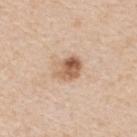Findings:
• notes: imaged on a skin check; not biopsied
• lesion diameter: about 3.5 mm
• lighting: white-light
• patient: female, aged around 50
• automated metrics: a border-irregularity rating of about 2/10 and peripheral color asymmetry of about 3.5
• anatomic site: the back
• image: ~15 mm crop, total-body skin-cancer survey